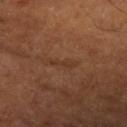{
  "biopsy_status": "not biopsied; imaged during a skin examination",
  "lighting": "cross-polarized",
  "lesion_size": {
    "long_diameter_mm_approx": 3.5
  },
  "patient": {
    "sex": "male",
    "age_approx": 65
  },
  "image": {
    "source": "total-body photography crop",
    "field_of_view_mm": 15
  },
  "site": "arm"
}